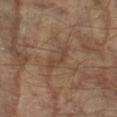| field | value |
|---|---|
| biopsy status | imaged on a skin check; not biopsied |
| patient | male, approximately 65 years of age |
| lesion diameter | ~3.5 mm (longest diameter) |
| imaging modality | ~15 mm tile from a whole-body skin photo |
| lighting | cross-polarized |
| anatomic site | the left forearm |